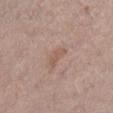Clinical impression:
Recorded during total-body skin imaging; not selected for excision or biopsy.
Image and clinical context:
An algorithmic analysis of the crop reported an average lesion color of about L≈56 a*≈18 b*≈26 (CIELAB), roughly 6 lightness units darker than nearby skin, and a normalized border contrast of about 5. The software also gave border irregularity of about 3.5 on a 0–10 scale, a within-lesion color-variation index near 1/10, and peripheral color asymmetry of about 0.5. It also reported a nevus-likeness score of about 0/100. On the right lower leg. A region of skin cropped from a whole-body photographic capture, roughly 15 mm wide. The lesion's longest dimension is about 2.5 mm. The tile uses white-light illumination. A female patient aged approximately 65.